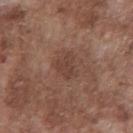biopsy_status: not biopsied; imaged during a skin examination
automated_metrics:
  cielab_L: 41
  cielab_a: 19
  cielab_b: 24
  vs_skin_darker_L: 7.0
  border_irregularity_0_10: 4.0
  color_variation_0_10: 1.5
  peripheral_color_asymmetry: 0.5
  nevus_likeness_0_100: 5
  lesion_detection_confidence_0_100: 100
site: chest
patient:
  sex: male
  age_approx: 75
image:
  source: total-body photography crop
  field_of_view_mm: 15
lesion_size:
  long_diameter_mm_approx: 3.0